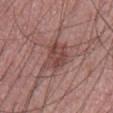biopsy status = catalogued during a skin exam; not biopsied
acquisition = ~15 mm tile from a whole-body skin photo
illumination = white-light illumination
location = the abdomen
size = ≈4 mm
subject = male, in their 60s